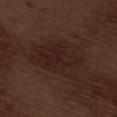Imaged during a routine full-body skin examination; the lesion was not biopsied and no histopathology is available. A male patient aged 68–72. Measured at roughly 7 mm in maximum diameter. Imaged with white-light lighting. Automated image analysis of the tile measured a footprint of about 24 mm². The analysis additionally found a border-irregularity index near 3.5/10. A 15 mm close-up tile from a total-body photography series done for melanoma screening. The lesion is on the right thigh.